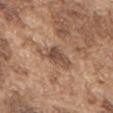Clinical impression:
Part of a total-body skin-imaging series; this lesion was reviewed on a skin check and was not flagged for biopsy.
Background:
A roughly 15 mm field-of-view crop from a total-body skin photograph. Located on the front of the torso. The patient is a male about 75 years old.